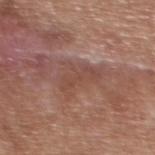Recorded during total-body skin imaging; not selected for excision or biopsy. A region of skin cropped from a whole-body photographic capture, roughly 15 mm wide. This is a white-light tile. The subject is a female aged 38 to 42. Located on the upper back. The lesion's longest dimension is about 5 mm.